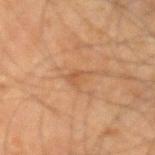Part of a total-body skin-imaging series; this lesion was reviewed on a skin check and was not flagged for biopsy. The lesion-visualizer software estimated an average lesion color of about L≈47 a*≈20 b*≈33 (CIELAB), about 6 CIELAB-L* units darker than the surrounding skin, and a lesion-to-skin contrast of about 5 (normalized; higher = more distinct). And it measured border irregularity of about 5 on a 0–10 scale and a within-lesion color-variation index near 0.5/10. Measured at roughly 2.5 mm in maximum diameter. This is a cross-polarized tile. A region of skin cropped from a whole-body photographic capture, roughly 15 mm wide. The subject is a male aged 63 to 67. The lesion is located on the left forearm.